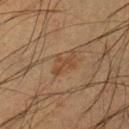Notes:
* workup — imaged on a skin check; not biopsied
* subject — male, aged 33 to 37
* image — 15 mm crop, total-body photography
* lighting — cross-polarized
* anatomic site — the left lower leg